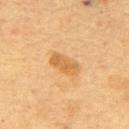{
  "biopsy_status": "not biopsied; imaged during a skin examination",
  "site": "upper back",
  "automated_metrics": {
    "area_mm2_approx": 6.0,
    "eccentricity": 0.9,
    "shape_asymmetry": 0.2,
    "cielab_L": 56,
    "cielab_a": 20,
    "cielab_b": 41,
    "vs_skin_darker_L": 9.0,
    "vs_skin_contrast_norm": 7.0
  },
  "image": {
    "source": "total-body photography crop",
    "field_of_view_mm": 15
  },
  "patient": {
    "sex": "female",
    "age_approx": 60
  }
}